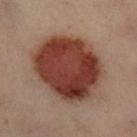Findings:
– biopsy status: catalogued during a skin exam; not biopsied
– lesion diameter: ~8 mm (longest diameter)
– patient: female, approximately 60 years of age
– image: ~15 mm crop, total-body skin-cancer survey
– location: the left leg